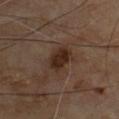Notes:
• notes · total-body-photography surveillance lesion; no biopsy
• patient · male, approximately 70 years of age
• lighting · cross-polarized illumination
• body site · the chest
• diameter · ≈4 mm
• imaging modality · ~15 mm crop, total-body skin-cancer survey